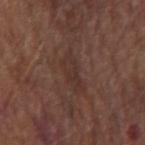Assessment: Imaged during a routine full-body skin examination; the lesion was not biopsied and no histopathology is available. Background: The lesion-visualizer software estimated a lesion area of about 7.5 mm², an eccentricity of roughly 0.9, and a symmetry-axis asymmetry near 0.35. The software also gave a lesion color around L≈32 a*≈18 b*≈22 in CIELAB and a normalized lesion–skin contrast near 5. The software also gave internal color variation of about 2 on a 0–10 scale. It also reported a classifier nevus-likeness of about 0/100 and a detector confidence of about 85 out of 100 that the crop contains a lesion. A male subject, aged 63–67. From the left forearm. Cropped from a whole-body photographic skin survey; the tile spans about 15 mm. Measured at roughly 5 mm in maximum diameter. The tile uses white-light illumination.